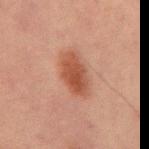{
  "biopsy_status": "not biopsied; imaged during a skin examination",
  "lesion_size": {
    "long_diameter_mm_approx": 4.5
  },
  "image": {
    "source": "total-body photography crop",
    "field_of_view_mm": 15
  },
  "patient": {
    "sex": "male",
    "age_approx": 65
  },
  "site": "abdomen"
}